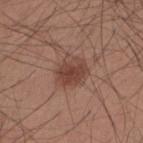Clinical impression: Recorded during total-body skin imaging; not selected for excision or biopsy. Acquisition and patient details: The lesion is located on the upper back. A 15 mm close-up tile from a total-body photography series done for melanoma screening. The total-body-photography lesion software estimated a lesion area of about 10 mm², an outline eccentricity of about 0.5 (0 = round, 1 = elongated), and a shape-asymmetry score of about 0.2 (0 = symmetric). Approximately 4 mm at its widest. A male patient, roughly 35 years of age.